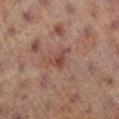The lesion was photographed on a routine skin check and not biopsied; there is no pathology result. On the left leg. A 15 mm crop from a total-body photograph taken for skin-cancer surveillance. The tile uses cross-polarized illumination. A female subject aged around 80. Measured at roughly 3 mm in maximum diameter.